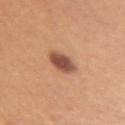Part of a total-body skin-imaging series; this lesion was reviewed on a skin check and was not flagged for biopsy.
A lesion tile, about 15 mm wide, cut from a 3D total-body photograph.
The patient is a female aged 23 to 27.
The lesion is located on the right upper arm.